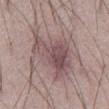Impression: The lesion was tiled from a total-body skin photograph and was not biopsied. Clinical summary: The tile uses white-light illumination. A male subject aged 48 to 52. This image is a 15 mm lesion crop taken from a total-body photograph. Located on the abdomen.